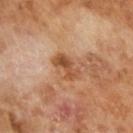Acquisition and patient details: An algorithmic analysis of the crop reported a mean CIELAB color near L≈49 a*≈23 b*≈35 and a normalized lesion–skin contrast near 8. And it measured a nevus-likeness score of about 0/100 and a detector confidence of about 100 out of 100 that the crop contains a lesion. The subject is a male approximately 65 years of age. A region of skin cropped from a whole-body photographic capture, roughly 15 mm wide. The tile uses cross-polarized illumination. Longest diameter approximately 3.5 mm.